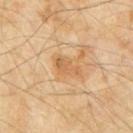imaging modality = total-body-photography crop, ~15 mm field of view | lighting = cross-polarized | anatomic site = the chest | subject = male, aged around 60.